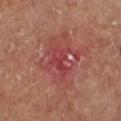follow-up = catalogued during a skin exam; not biopsied | imaging modality = ~15 mm tile from a whole-body skin photo | patient = male, aged approximately 65 | lighting = cross-polarized | anatomic site = the left lower leg | automated lesion analysis = a footprint of about 6.5 mm², an eccentricity of roughly 0.8, and two-axis asymmetry of about 0.4; a lesion color around L≈42 a*≈34 b*≈23 in CIELAB; border irregularity of about 6 on a 0–10 scale, internal color variation of about 2.5 on a 0–10 scale, and a peripheral color-asymmetry measure near 1 | lesion size = about 4 mm.